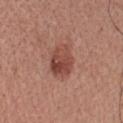workup: catalogued during a skin exam; not biopsied | image source: 15 mm crop, total-body photography | diameter: about 4 mm | subject: male, aged approximately 50 | location: the chest | tile lighting: white-light illumination.